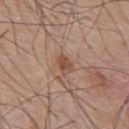No biopsy was performed on this lesion — it was imaged during a full skin examination and was not determined to be concerning. Longest diameter approximately 3 mm. Located on the back. Captured under white-light illumination. The patient is a male aged 53–57. A region of skin cropped from a whole-body photographic capture, roughly 15 mm wide.